The tile uses cross-polarized illumination. A male patient, aged around 75. On the lower back. The recorded lesion diameter is about 6.5 mm. A 15 mm close-up tile from a total-body photography series done for melanoma screening. The total-body-photography lesion software estimated a mean CIELAB color near L≈41 a*≈21 b*≈27, a lesion–skin lightness drop of about 8, and a normalized lesion–skin contrast near 7:
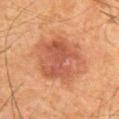Histopathology of the biopsied lesion showed a dysplastic (Clark) nevus.